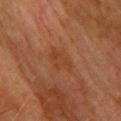The lesion was photographed on a routine skin check and not biopsied; there is no pathology result. About 3.5 mm across. Imaged with cross-polarized lighting. A 15 mm close-up tile from a total-body photography series done for melanoma screening. The subject is a female approximately 55 years of age. Located on the upper back. Automated image analysis of the tile measured border irregularity of about 4.5 on a 0–10 scale, a within-lesion color-variation index near 2/10, and a peripheral color-asymmetry measure near 0.5. The software also gave a classifier nevus-likeness of about 0/100 and a lesion-detection confidence of about 100/100.